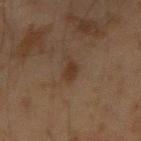The lesion was tiled from a total-body skin photograph and was not biopsied. This is a cross-polarized tile. A roughly 15 mm field-of-view crop from a total-body skin photograph. A male patient aged around 45. From the left forearm. The lesion-visualizer software estimated a lesion color around L≈27 a*≈13 b*≈23 in CIELAB and a normalized lesion–skin contrast near 7. And it measured a border-irregularity index near 3/10, internal color variation of about 1 on a 0–10 scale, and peripheral color asymmetry of about 0.5.